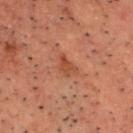Clinical impression: Recorded during total-body skin imaging; not selected for excision or biopsy. Clinical summary: From the head or neck. The recorded lesion diameter is about 2.5 mm. A 15 mm crop from a total-body photograph taken for skin-cancer surveillance. A male subject roughly 50 years of age.